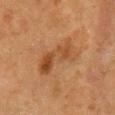Impression:
Recorded during total-body skin imaging; not selected for excision or biopsy.
Context:
The patient is a female aged around 50. Captured under cross-polarized illumination. A close-up tile cropped from a whole-body skin photograph, about 15 mm across. The recorded lesion diameter is about 5.5 mm. Located on the chest.